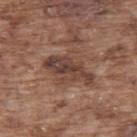Assessment:
Captured during whole-body skin photography for melanoma surveillance; the lesion was not biopsied.
Acquisition and patient details:
This is a white-light tile. Longest diameter approximately 5.5 mm. Automated image analysis of the tile measured an area of roughly 8.5 mm² and an outline eccentricity of about 0.95 (0 = round, 1 = elongated). The analysis additionally found a classifier nevus-likeness of about 0/100. The lesion is located on the back. Cropped from a whole-body photographic skin survey; the tile spans about 15 mm. A male patient, aged 73 to 77.